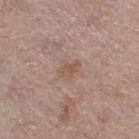<case>
  <biopsy_status>not biopsied; imaged during a skin examination</biopsy_status>
  <lesion_size>
    <long_diameter_mm_approx>2.5</long_diameter_mm_approx>
  </lesion_size>
  <lighting>white-light</lighting>
  <patient>
    <sex>female</sex>
    <age_approx>65</age_approx>
  </patient>
  <site>leg</site>
  <image>
    <source>total-body photography crop</source>
    <field_of_view_mm>15</field_of_view_mm>
  </image>
</case>